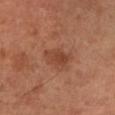No biopsy was performed on this lesion — it was imaged during a full skin examination and was not determined to be concerning. A roughly 15 mm field-of-view crop from a total-body skin photograph. The lesion is located on the left lower leg. A male patient, in their mid- to late 60s. Approximately 3.5 mm at its widest. This is a cross-polarized tile.